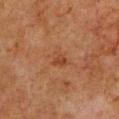follow-up — no biopsy performed (imaged during a skin exam); patient — male, aged approximately 80; illumination — cross-polarized; site — the front of the torso; image — ~15 mm tile from a whole-body skin photo.